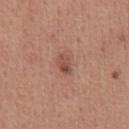The subject is a male about 45 years old. Cropped from a total-body skin-imaging series; the visible field is about 15 mm. The total-body-photography lesion software estimated an average lesion color of about L≈49 a*≈23 b*≈26 (CIELAB), roughly 10 lightness units darker than nearby skin, and a lesion-to-skin contrast of about 7 (normalized; higher = more distinct). The analysis additionally found a border-irregularity index near 2.5/10, a within-lesion color-variation index near 5.5/10, and peripheral color asymmetry of about 2.5. The software also gave a classifier nevus-likeness of about 40/100 and lesion-presence confidence of about 100/100. Approximately 2.5 mm at its widest. Captured under white-light illumination. The lesion is on the chest.